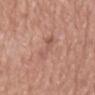Impression: Captured during whole-body skin photography for melanoma surveillance; the lesion was not biopsied. Clinical summary: A male subject, in their mid-70s. A 15 mm close-up extracted from a 3D total-body photography capture. About 4 mm across. This is a white-light tile. From the mid back.